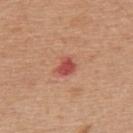Imaged during a routine full-body skin examination; the lesion was not biopsied and no histopathology is available. Automated image analysis of the tile measured a lesion area of about 4 mm², a shape eccentricity near 0.55, and two-axis asymmetry of about 0.25. The analysis additionally found an average lesion color of about L≈51 a*≈32 b*≈29 (CIELAB), about 12 CIELAB-L* units darker than the surrounding skin, and a normalized lesion–skin contrast near 8.5. The software also gave a color-variation rating of about 2/10. It also reported a classifier nevus-likeness of about 0/100 and a lesion-detection confidence of about 100/100. The recorded lesion diameter is about 2.5 mm. The tile uses white-light illumination. Cropped from a whole-body photographic skin survey; the tile spans about 15 mm. The subject is a female in their mid- to late 40s. Located on the upper back.